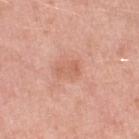The patient is a female about 70 years old. The lesion's longest dimension is about 2.5 mm. The lesion is on the left thigh. Cropped from a total-body skin-imaging series; the visible field is about 15 mm. Imaged with white-light lighting.